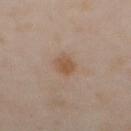<case>
  <biopsy_status>not biopsied; imaged during a skin examination</biopsy_status>
  <image>
    <source>total-body photography crop</source>
    <field_of_view_mm>15</field_of_view_mm>
  </image>
  <site>abdomen</site>
  <patient>
    <sex>male</sex>
    <age_approx>65</age_approx>
  </patient>
</case>